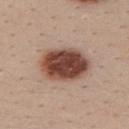This lesion was catalogued during total-body skin photography and was not selected for biopsy.
Imaged with white-light lighting.
The lesion is located on the back.
The subject is a female roughly 25 years of age.
Cropped from a whole-body photographic skin survey; the tile spans about 15 mm.
Longest diameter approximately 6 mm.